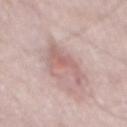No biopsy was performed on this lesion — it was imaged during a full skin examination and was not determined to be concerning.
The tile uses white-light illumination.
From the mid back.
A male subject in their 50s.
A 15 mm close-up tile from a total-body photography series done for melanoma screening.
The recorded lesion diameter is about 5.5 mm.